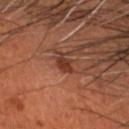Clinical impression: Imaged during a routine full-body skin examination; the lesion was not biopsied and no histopathology is available. Image and clinical context: Captured under cross-polarized illumination. On the head or neck. A roughly 15 mm field-of-view crop from a total-body skin photograph. The lesion-visualizer software estimated border irregularity of about 2 on a 0–10 scale, internal color variation of about 1 on a 0–10 scale, and a peripheral color-asymmetry measure near 0.5. The subject is a male aged 53 to 57.